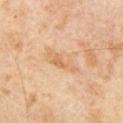Q: What kind of image is this?
A: 15 mm crop, total-body photography
Q: Patient demographics?
A: male, in their mid-60s
Q: Automated lesion metrics?
A: a footprint of about 7 mm², an outline eccentricity of about 0.9 (0 = round, 1 = elongated), and a shape-asymmetry score of about 0.3 (0 = symmetric); a lesion–skin lightness drop of about 7 and a normalized lesion–skin contrast near 5.5
Q: Illumination type?
A: cross-polarized
Q: What is the lesion's diameter?
A: ~5 mm (longest diameter)